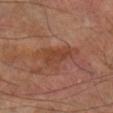Clinical impression:
This lesion was catalogued during total-body skin photography and was not selected for biopsy.